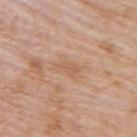Clinical impression: Recorded during total-body skin imaging; not selected for excision or biopsy. Acquisition and patient details: This is a white-light tile. The total-body-photography lesion software estimated a lesion color around L≈59 a*≈20 b*≈33 in CIELAB, about 6 CIELAB-L* units darker than the surrounding skin, and a normalized lesion–skin contrast near 4.5. And it measured a lesion-detection confidence of about 100/100. A 15 mm crop from a total-body photograph taken for skin-cancer surveillance. Located on the back. The patient is a male aged 73–77. The lesion's longest dimension is about 3 mm.